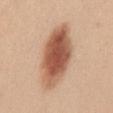Q: What did automated image analysis measure?
A: a footprint of about 26 mm², an outline eccentricity of about 0.9 (0 = round, 1 = elongated), and a symmetry-axis asymmetry near 0.15; a lesion color around L≈56 a*≈23 b*≈32 in CIELAB, about 17 CIELAB-L* units darker than the surrounding skin, and a lesion-to-skin contrast of about 10.5 (normalized; higher = more distinct); an automated nevus-likeness rating near 100 out of 100 and lesion-presence confidence of about 100/100
Q: What is the lesion's diameter?
A: ≈8.5 mm
Q: Lesion location?
A: the mid back
Q: Illumination type?
A: white-light
Q: Who is the patient?
A: female, in their 30s
Q: What is the imaging modality?
A: ~15 mm crop, total-body skin-cancer survey